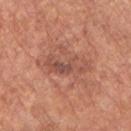Impression:
This lesion was catalogued during total-body skin photography and was not selected for biopsy.
Acquisition and patient details:
An algorithmic analysis of the crop reported a footprint of about 7.5 mm², an outline eccentricity of about 0.9 (0 = round, 1 = elongated), and a symmetry-axis asymmetry near 0.5. The software also gave a normalized lesion–skin contrast near 6.5. It also reported an automated nevus-likeness rating near 5 out of 100 and a lesion-detection confidence of about 100/100. About 5 mm across. Imaged with white-light lighting. A male patient, aged 63–67. The lesion is on the chest. Cropped from a whole-body photographic skin survey; the tile spans about 15 mm.